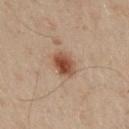  biopsy_status: not biopsied; imaged during a skin examination
  site: arm
  image:
    source: total-body photography crop
    field_of_view_mm: 15
  patient:
    sex: male
    age_approx: 50